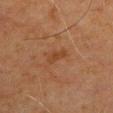<lesion>
  <biopsy_status>not biopsied; imaged during a skin examination</biopsy_status>
  <patient>
    <sex>male</sex>
    <age_approx>70</age_approx>
  </patient>
  <site>arm</site>
  <image>
    <source>total-body photography crop</source>
    <field_of_view_mm>15</field_of_view_mm>
  </image>
  <automated_metrics>
    <area_mm2_approx>3.5</area_mm2_approx>
    <eccentricity>0.85</eccentricity>
    <shape_asymmetry>0.35</shape_asymmetry>
    <color_variation_0_10>1.0</color_variation_0_10>
    <peripheral_color_asymmetry>0.5</peripheral_color_asymmetry>
  </automated_metrics>
</lesion>